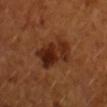This lesion was catalogued during total-body skin photography and was not selected for biopsy. The lesion is located on the right forearm. An algorithmic analysis of the crop reported a lesion color around L≈28 a*≈24 b*≈30 in CIELAB, a lesion–skin lightness drop of about 11, and a normalized border contrast of about 10.5. And it measured a within-lesion color-variation index near 8/10 and a peripheral color-asymmetry measure near 3. It also reported an automated nevus-likeness rating near 85 out of 100 and lesion-presence confidence of about 100/100. A female patient, approximately 55 years of age. About 5.5 mm across. A lesion tile, about 15 mm wide, cut from a 3D total-body photograph. Captured under cross-polarized illumination.